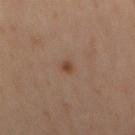biopsy status — imaged on a skin check; not biopsied | subject — male, aged approximately 65 | lesion size — ~2 mm (longest diameter) | acquisition — ~15 mm tile from a whole-body skin photo | tile lighting — cross-polarized illumination | anatomic site — the mid back | automated metrics — a lesion color around L≈42 a*≈18 b*≈29 in CIELAB and a lesion–skin lightness drop of about 8; a peripheral color-asymmetry measure near 0; a classifier nevus-likeness of about 90/100 and lesion-presence confidence of about 100/100.